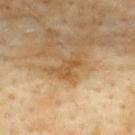{"biopsy_status": "not biopsied; imaged during a skin examination", "automated_metrics": {"area_mm2_approx": 5.5, "shape_asymmetry": 0.5, "cielab_L": 49, "cielab_a": 17, "cielab_b": 37, "vs_skin_darker_L": 7.0, "vs_skin_contrast_norm": 6.0, "nevus_likeness_0_100": 0}, "lesion_size": {"long_diameter_mm_approx": 4.0}, "site": "upper back", "lighting": "cross-polarized", "image": {"source": "total-body photography crop", "field_of_view_mm": 15}, "patient": {"sex": "female", "age_approx": 60}}